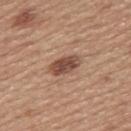Captured during whole-body skin photography for melanoma surveillance; the lesion was not biopsied. From the upper back. A region of skin cropped from a whole-body photographic capture, roughly 15 mm wide. A female patient approximately 55 years of age. The lesion's longest dimension is about 4 mm. This is a white-light tile.